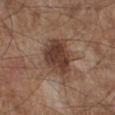Findings:
– follow-up: no biopsy performed (imaged during a skin exam)
– patient: male, aged approximately 55
– location: the right lower leg
– image: 15 mm crop, total-body photography